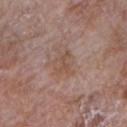Impression: The lesion was photographed on a routine skin check and not biopsied; there is no pathology result. Context: This image is a 15 mm lesion crop taken from a total-body photograph. Captured under white-light illumination. The recorded lesion diameter is about 3 mm. From the right upper arm. A female patient, aged 68 to 72.